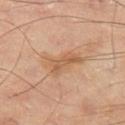biopsy_status: not biopsied; imaged during a skin examination
patient:
  sex: male
  age_approx: 65
image:
  source: total-body photography crop
  field_of_view_mm: 15
site: leg
lesion_size:
  long_diameter_mm_approx: 4.5